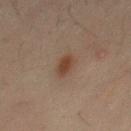This lesion was catalogued during total-body skin photography and was not selected for biopsy. Cropped from a total-body skin-imaging series; the visible field is about 15 mm. On the mid back. The lesion-visualizer software estimated an area of roughly 4.5 mm², a shape eccentricity near 0.9, and a shape-asymmetry score of about 0.35 (0 = symmetric). The recorded lesion diameter is about 3.5 mm. This is a cross-polarized tile. The patient is a male aged around 50.